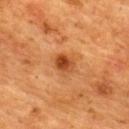Clinical impression: This lesion was catalogued during total-body skin photography and was not selected for biopsy. Image and clinical context: A female subject aged 53 to 57. A close-up tile cropped from a whole-body skin photograph, about 15 mm across. Measured at roughly 3 mm in maximum diameter. Automated image analysis of the tile measured an area of roughly 5.5 mm², an eccentricity of roughly 0.55, and two-axis asymmetry of about 0.25. And it measured a lesion–skin lightness drop of about 10 and a normalized lesion–skin contrast near 8.5. The lesion is located on the upper back. Captured under cross-polarized illumination.